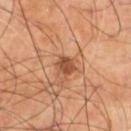follow-up: catalogued during a skin exam; not biopsied
imaging modality: 15 mm crop, total-body photography
illumination: cross-polarized illumination
body site: the left lower leg
lesion diameter: ~3 mm (longest diameter)
subject: male, aged 68–72